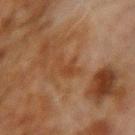Recorded during total-body skin imaging; not selected for excision or biopsy. Longest diameter approximately 3.5 mm. The subject is a male roughly 70 years of age. This is a cross-polarized tile. The lesion-visualizer software estimated a border-irregularity rating of about 7.5/10, internal color variation of about 0.5 on a 0–10 scale, and peripheral color asymmetry of about 0.5. The analysis additionally found an automated nevus-likeness rating near 0 out of 100 and lesion-presence confidence of about 100/100. Located on the right upper arm. Cropped from a total-body skin-imaging series; the visible field is about 15 mm.